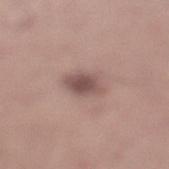Assessment:
No biopsy was performed on this lesion — it was imaged during a full skin examination and was not determined to be concerning.
Background:
The lesion is located on the left lower leg. Approximately 3 mm at its widest. Imaged with white-light lighting. A close-up tile cropped from a whole-body skin photograph, about 15 mm across. A male patient, aged 53 to 57.